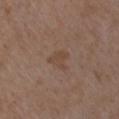Imaged during a routine full-body skin examination; the lesion was not biopsied and no histopathology is available.
The recorded lesion diameter is about 3 mm.
The lesion-visualizer software estimated an average lesion color of about L≈44 a*≈16 b*≈26 (CIELAB), roughly 5 lightness units darker than nearby skin, and a normalized border contrast of about 5.5. It also reported a border-irregularity index near 5/10 and a color-variation rating of about 1.5/10. The analysis additionally found a nevus-likeness score of about 0/100 and a lesion-detection confidence of about 100/100.
A male subject in their 50s.
A 15 mm close-up extracted from a 3D total-body photography capture.
The lesion is located on the left upper arm.
Imaged with white-light lighting.